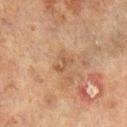This lesion was catalogued during total-body skin photography and was not selected for biopsy.
Automated tile analysis of the lesion measured a footprint of about 3 mm², a shape eccentricity near 0.75, and a symmetry-axis asymmetry near 0.55. And it measured a border-irregularity index near 7/10 and a within-lesion color-variation index near 0/10.
Imaged with cross-polarized lighting.
The subject is a male about 70 years old.
Cropped from a total-body skin-imaging series; the visible field is about 15 mm.
From the leg.